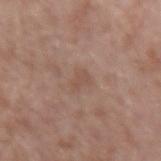Impression: Part of a total-body skin-imaging series; this lesion was reviewed on a skin check and was not flagged for biopsy. Acquisition and patient details: The lesion is on the left forearm. A male patient aged approximately 65. A roughly 15 mm field-of-view crop from a total-body skin photograph.